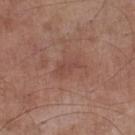Captured during whole-body skin photography for melanoma surveillance; the lesion was not biopsied. The lesion is on the left lower leg. A male subject aged 53 to 57. A region of skin cropped from a whole-body photographic capture, roughly 15 mm wide. Imaged with white-light lighting.